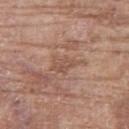Q: Was a biopsy performed?
A: catalogued during a skin exam; not biopsied
Q: Automated lesion metrics?
A: roughly 7 lightness units darker than nearby skin and a normalized border contrast of about 5.5; a border-irregularity index near 4.5/10, a color-variation rating of about 2/10, and a peripheral color-asymmetry measure near 1
Q: Lesion size?
A: ~4 mm (longest diameter)
Q: Lesion location?
A: the leg
Q: Illumination type?
A: white-light
Q: What kind of image is this?
A: total-body-photography crop, ~15 mm field of view
Q: What are the patient's age and sex?
A: female, approximately 75 years of age